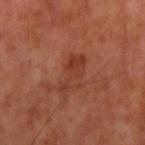follow-up: catalogued during a skin exam; not biopsied | imaging modality: ~15 mm crop, total-body skin-cancer survey | lighting: cross-polarized | automated metrics: an outline eccentricity of about 0.9 (0 = round, 1 = elongated) and a shape-asymmetry score of about 0.5 (0 = symmetric); a lesion color around L≈37 a*≈27 b*≈31 in CIELAB and a normalized lesion–skin contrast near 6; a nevus-likeness score of about 0/100 and lesion-presence confidence of about 100/100 | lesion diameter: about 5 mm | site: the arm | patient: male, in their mid-60s.